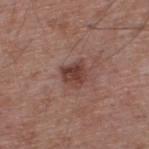Imaged during a routine full-body skin examination; the lesion was not biopsied and no histopathology is available.
Automated image analysis of the tile measured a lesion color around L≈41 a*≈21 b*≈23 in CIELAB, roughly 11 lightness units darker than nearby skin, and a normalized lesion–skin contrast near 8.5. It also reported a nevus-likeness score of about 50/100 and a lesion-detection confidence of about 100/100.
The tile uses white-light illumination.
A male patient, aged 53–57.
From the back.
The lesion's longest dimension is about 3 mm.
Cropped from a whole-body photographic skin survey; the tile spans about 15 mm.